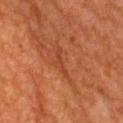biopsy_status: not biopsied; imaged during a skin examination
patient:
  sex: female
  age_approx: 65
site: upper back
lighting: cross-polarized
image:
  source: total-body photography crop
  field_of_view_mm: 15
automated_metrics:
  cielab_L: 42
  cielab_a: 30
  cielab_b: 36
  vs_skin_darker_L: 6.0
  vs_skin_contrast_norm: 5.0
  nevus_likeness_0_100: 0
  lesion_detection_confidence_0_100: 50